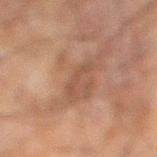Impression: No biopsy was performed on this lesion — it was imaged during a full skin examination and was not determined to be concerning. Clinical summary: The tile uses cross-polarized illumination. The lesion-visualizer software estimated an area of roughly 3 mm², an outline eccentricity of about 0.95 (0 = round, 1 = elongated), and two-axis asymmetry of about 0.55. The analysis additionally found a lesion-detection confidence of about 100/100. The patient is a male aged 58–62. A region of skin cropped from a whole-body photographic capture, roughly 15 mm wide. Longest diameter approximately 3.5 mm. From the left leg.